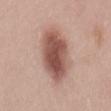Findings:
• image — ~15 mm crop, total-body skin-cancer survey
• site — the back
• size — about 7.5 mm
• TBP lesion metrics — an area of roughly 23 mm², a shape eccentricity near 0.85, and a shape-asymmetry score of about 0.15 (0 = symmetric); an average lesion color of about L≈53 a*≈21 b*≈25 (CIELAB) and roughly 15 lightness units darker than nearby skin; a within-lesion color-variation index near 6/10 and radial color variation of about 2
• subject — male, roughly 25 years of age